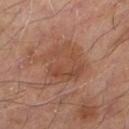Clinical impression:
The lesion was tiled from a total-body skin photograph and was not biopsied.
Clinical summary:
Cropped from a whole-body photographic skin survey; the tile spans about 15 mm. The patient is a male in their mid- to late 50s. Longest diameter approximately 5 mm. Automated tile analysis of the lesion measured a border-irregularity rating of about 4/10 and a peripheral color-asymmetry measure near 1. The software also gave a nevus-likeness score of about 0/100 and lesion-presence confidence of about 100/100. The tile uses cross-polarized illumination. On the left thigh.